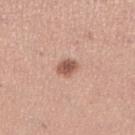Clinical impression: The lesion was photographed on a routine skin check and not biopsied; there is no pathology result. Clinical summary: Measured at roughly 2.5 mm in maximum diameter. An algorithmic analysis of the crop reported a lesion area of about 4 mm². The analysis additionally found border irregularity of about 1.5 on a 0–10 scale and a within-lesion color-variation index near 2/10. A female subject aged 28–32. Imaged with white-light lighting. On the right lower leg. Cropped from a whole-body photographic skin survey; the tile spans about 15 mm.